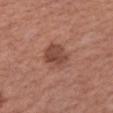No biopsy was performed on this lesion — it was imaged during a full skin examination and was not determined to be concerning. A lesion tile, about 15 mm wide, cut from a 3D total-body photograph. An algorithmic analysis of the crop reported an average lesion color of about L≈46 a*≈23 b*≈27 (CIELAB), a lesion–skin lightness drop of about 10, and a normalized lesion–skin contrast near 7.5. The software also gave a border-irregularity index near 2/10, a color-variation rating of about 3/10, and radial color variation of about 1. On the arm. Imaged with white-light lighting. Approximately 4 mm at its widest. A female subject roughly 60 years of age.